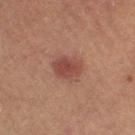Cropped from a total-body skin-imaging series; the visible field is about 15 mm. The tile uses cross-polarized illumination. A female patient, aged 58 to 62. Approximately 3.5 mm at its widest. The lesion is located on the leg.